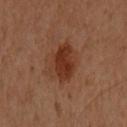follow-up = imaged on a skin check; not biopsied | lighting = cross-polarized | subject = female, roughly 60 years of age | lesion size = ~4.5 mm (longest diameter) | site = the front of the torso | image source = total-body-photography crop, ~15 mm field of view.